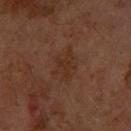Q: Was this lesion biopsied?
A: catalogued during a skin exam; not biopsied
Q: What is the lesion's diameter?
A: ~4.5 mm (longest diameter)
Q: How was the tile lit?
A: cross-polarized
Q: What is the anatomic site?
A: the right upper arm
Q: What did automated image analysis measure?
A: a footprint of about 9.5 mm² and two-axis asymmetry of about 0.25; about 4 CIELAB-L* units darker than the surrounding skin and a lesion-to-skin contrast of about 5 (normalized; higher = more distinct)
Q: What are the patient's age and sex?
A: female, aged around 60
Q: How was this image acquired?
A: 15 mm crop, total-body photography